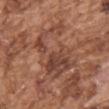Q: Was a biopsy performed?
A: total-body-photography surveillance lesion; no biopsy
Q: Illumination type?
A: white-light illumination
Q: How was this image acquired?
A: ~15 mm crop, total-body skin-cancer survey
Q: How large is the lesion?
A: about 6 mm
Q: What is the anatomic site?
A: the upper back
Q: Patient demographics?
A: male, aged 73–77